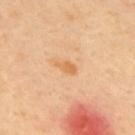Part of a total-body skin-imaging series; this lesion was reviewed on a skin check and was not flagged for biopsy.
This is a cross-polarized tile.
A roughly 15 mm field-of-view crop from a total-body skin photograph.
A male patient, in their 40s.
The lesion's longest dimension is about 2.5 mm.
The lesion is located on the upper back.
Automated image analysis of the tile measured a lesion area of about 2.5 mm², an eccentricity of roughly 0.85, and a shape-asymmetry score of about 0.35 (0 = symmetric). The software also gave a lesion color around L≈66 a*≈23 b*≈44 in CIELAB, about 8 CIELAB-L* units darker than the surrounding skin, and a normalized border contrast of about 6.5. And it measured a border-irregularity index near 3/10, a within-lesion color-variation index near 0.5/10, and peripheral color asymmetry of about 0.